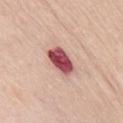Assessment: The lesion was tiled from a total-body skin photograph and was not biopsied. Background: Imaged with white-light lighting. Approximately 4 mm at its widest. The patient is a female aged approximately 70. Located on the chest. This image is a 15 mm lesion crop taken from a total-body photograph.